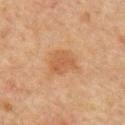Clinical impression:
Recorded during total-body skin imaging; not selected for excision or biopsy.
Background:
A roughly 15 mm field-of-view crop from a total-body skin photograph. The subject is a male approximately 65 years of age. On the back.